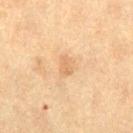Part of a total-body skin-imaging series; this lesion was reviewed on a skin check and was not flagged for biopsy.
Automated image analysis of the tile measured a footprint of about 4 mm² and an eccentricity of roughly 0.7. The analysis additionally found a lesion color around L≈60 a*≈17 b*≈35 in CIELAB, about 7 CIELAB-L* units darker than the surrounding skin, and a normalized lesion–skin contrast near 4.5. It also reported radial color variation of about 0.5.
A 15 mm close-up tile from a total-body photography series done for melanoma screening.
Approximately 2.5 mm at its widest.
A female patient in their 40s.
Captured under cross-polarized illumination.
The lesion is located on the right thigh.